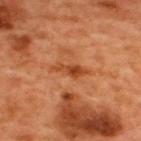Clinical impression:
Imaged during a routine full-body skin examination; the lesion was not biopsied and no histopathology is available.
Clinical summary:
The tile uses cross-polarized illumination. Approximately 4.5 mm at its widest. Cropped from a whole-body photographic skin survey; the tile spans about 15 mm. An algorithmic analysis of the crop reported an area of roughly 5 mm² and a shape-asymmetry score of about 0.3 (0 = symmetric). The software also gave a color-variation rating of about 3/10. The lesion is located on the upper back. A male patient aged 48 to 52.